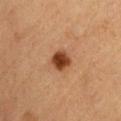Imaged during a routine full-body skin examination; the lesion was not biopsied and no histopathology is available.
The tile uses cross-polarized illumination.
A male subject, aged approximately 85 or older.
Longest diameter approximately 2.5 mm.
The lesion is located on the abdomen.
Automated image analysis of the tile measured a border-irregularity rating of about 1/10, a color-variation rating of about 3/10, and peripheral color asymmetry of about 1. And it measured an automated nevus-likeness rating near 100 out of 100 and a lesion-detection confidence of about 100/100.
Cropped from a total-body skin-imaging series; the visible field is about 15 mm.